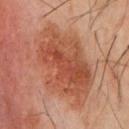No biopsy was performed on this lesion — it was imaged during a full skin examination and was not determined to be concerning. Located on the chest. A 15 mm crop from a total-body photograph taken for skin-cancer surveillance. The patient is a male aged 58 to 62. This is a cross-polarized tile.